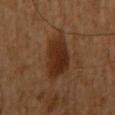This is a cross-polarized tile.
The subject is a male approximately 60 years of age.
The recorded lesion diameter is about 4.5 mm.
The lesion is located on the left upper arm.
The lesion-visualizer software estimated a lesion color around L≈25 a*≈18 b*≈26 in CIELAB and a lesion–skin lightness drop of about 9. And it measured border irregularity of about 2.5 on a 0–10 scale and a peripheral color-asymmetry measure near 1.
Cropped from a whole-body photographic skin survey; the tile spans about 15 mm.